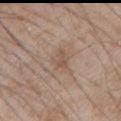Impression:
The lesion was tiled from a total-body skin photograph and was not biopsied.
Background:
Cropped from a whole-body photographic skin survey; the tile spans about 15 mm. The lesion is located on the front of the torso. A male patient aged around 65.